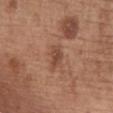Q: Was a biopsy performed?
A: total-body-photography surveillance lesion; no biopsy
Q: What is the anatomic site?
A: the chest
Q: Lesion size?
A: ~3 mm (longest diameter)
Q: Patient demographics?
A: female, approximately 55 years of age
Q: What lighting was used for the tile?
A: white-light
Q: What kind of image is this?
A: total-body-photography crop, ~15 mm field of view